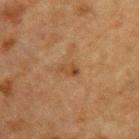{
  "patient": {
    "sex": "male",
    "age_approx": 50
  },
  "lighting": "cross-polarized",
  "site": "upper back",
  "image": {
    "source": "total-body photography crop",
    "field_of_view_mm": 15
  },
  "automated_metrics": {
    "border_irregularity_0_10": 2.5,
    "color_variation_0_10": 3.0,
    "nevus_likeness_0_100": 10
  }
}